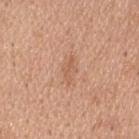• biopsy status · imaged on a skin check; not biopsied
• image · total-body-photography crop, ~15 mm field of view
• lighting · white-light illumination
• subject · male, in their 40s
• anatomic site · the head or neck
• lesion size · ~3 mm (longest diameter)